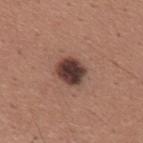Clinical impression:
This lesion was catalogued during total-body skin photography and was not selected for biopsy.
Clinical summary:
The total-body-photography lesion software estimated a lesion color around L≈38 a*≈19 b*≈21 in CIELAB and a lesion-to-skin contrast of about 13.5 (normalized; higher = more distinct). The software also gave border irregularity of about 1.5 on a 0–10 scale and internal color variation of about 5 on a 0–10 scale. The software also gave a nevus-likeness score of about 45/100 and lesion-presence confidence of about 100/100. This is a white-light tile. Located on the mid back. The patient is a male about 45 years old. A region of skin cropped from a whole-body photographic capture, roughly 15 mm wide. The recorded lesion diameter is about 3.5 mm.